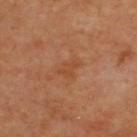- patient — in their mid-60s
- anatomic site — the upper back
- lesion diameter — ~3 mm (longest diameter)
- image source — ~15 mm crop, total-body skin-cancer survey